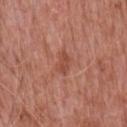biopsy_status: not biopsied; imaged during a skin examination
lesion_size:
  long_diameter_mm_approx: 3.0
image:
  source: total-body photography crop
  field_of_view_mm: 15
site: front of the torso
automated_metrics:
  border_irregularity_0_10: 3.0
  color_variation_0_10: 2.0
patient:
  sex: male
  age_approx: 65
lighting: white-light